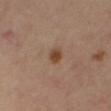workup = total-body-photography surveillance lesion; no biopsy | lighting = cross-polarized illumination | patient = female, approximately 50 years of age | TBP lesion metrics = a border-irregularity rating of about 1.5/10 and a color-variation rating of about 2/10 | acquisition = total-body-photography crop, ~15 mm field of view | anatomic site = the right thigh | size = ≈2 mm.